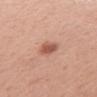workup: no biopsy performed (imaged during a skin exam)
tile lighting: white-light
patient: female, aged 43 to 47
diameter: ≈2.5 mm
site: the right upper arm
imaging modality: 15 mm crop, total-body photography
image-analysis metrics: an area of roughly 4 mm² and a shape-asymmetry score of about 0.25 (0 = symmetric); an average lesion color of about L≈55 a*≈25 b*≈29 (CIELAB) and a lesion–skin lightness drop of about 12; a border-irregularity rating of about 2.5/10, a color-variation rating of about 2.5/10, and a peripheral color-asymmetry measure near 1; a classifier nevus-likeness of about 95/100